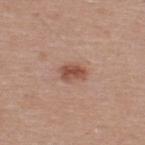Q: Is there a histopathology result?
A: total-body-photography surveillance lesion; no biopsy
Q: How was this image acquired?
A: total-body-photography crop, ~15 mm field of view
Q: Patient demographics?
A: male, aged 38 to 42
Q: What did automated image analysis measure?
A: a mean CIELAB color near L≈50 a*≈23 b*≈28, a lesion–skin lightness drop of about 12, and a normalized border contrast of about 8.5; an automated nevus-likeness rating near 90 out of 100 and lesion-presence confidence of about 100/100
Q: What is the lesion's diameter?
A: about 3 mm
Q: How was the tile lit?
A: white-light
Q: What is the anatomic site?
A: the upper back